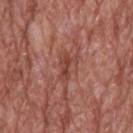workup — imaged on a skin check; not biopsied
patient — male, aged 68–72
tile lighting — white-light illumination
imaging modality — ~15 mm crop, total-body skin-cancer survey
automated metrics — an area of roughly 6 mm² and two-axis asymmetry of about 0.5
body site — the back
diameter — ~4 mm (longest diameter)